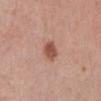The subject is a female aged 63–67.
A roughly 15 mm field-of-view crop from a total-body skin photograph.
Imaged with white-light lighting.
The lesion is on the left lower leg.
The lesion-visualizer software estimated an area of roughly 5 mm², an eccentricity of roughly 0.7, and a symmetry-axis asymmetry near 0.25.
Longest diameter approximately 3 mm.